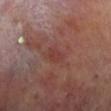image=15 mm crop, total-body photography | anatomic site=the leg | illumination=cross-polarized | automated metrics=an area of roughly 3.5 mm², a shape eccentricity near 0.7, and two-axis asymmetry of about 0.3; a normalized lesion–skin contrast near 5; a classifier nevus-likeness of about 0/100 and a detector confidence of about 100 out of 100 that the crop contains a lesion | lesion diameter=≈2.5 mm | patient=male, about 70 years old.